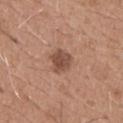follow-up = no biopsy performed (imaged during a skin exam) | image = total-body-photography crop, ~15 mm field of view | patient = male, about 65 years old | location = the chest.